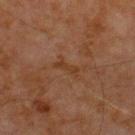A male subject aged around 70.
On the chest.
A 15 mm close-up tile from a total-body photography series done for melanoma screening.